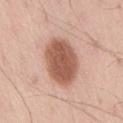Impression: Part of a total-body skin-imaging series; this lesion was reviewed on a skin check and was not flagged for biopsy. Clinical summary: An algorithmic analysis of the crop reported a footprint of about 20 mm², an eccentricity of roughly 0.7, and two-axis asymmetry of about 0.15. It also reported a mean CIELAB color near L≈56 a*≈23 b*≈29. The analysis additionally found an automated nevus-likeness rating near 100 out of 100. A roughly 15 mm field-of-view crop from a total-body skin photograph. Located on the lower back. The lesion's longest dimension is about 6 mm. A male patient, in their mid-50s. The tile uses white-light illumination.